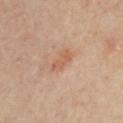Q: Was this lesion biopsied?
A: no biopsy performed (imaged during a skin exam)
Q: What is the lesion's diameter?
A: ≈3.5 mm
Q: Illumination type?
A: cross-polarized illumination
Q: Who is the patient?
A: aged approximately 55
Q: Where on the body is the lesion?
A: the chest
Q: What kind of image is this?
A: total-body-photography crop, ~15 mm field of view